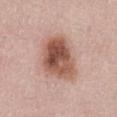Imaged during a routine full-body skin examination; the lesion was not biopsied and no histopathology is available. Approximately 6.5 mm at its widest. Imaged with white-light lighting. This image is a 15 mm lesion crop taken from a total-body photograph. The patient is a female in their mid-60s. On the front of the torso.